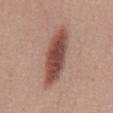The lesion was photographed on a routine skin check and not biopsied; there is no pathology result. The tile uses white-light illumination. This image is a 15 mm lesion crop taken from a total-body photograph. Located on the back. A female patient in their mid- to late 40s. The lesion-visualizer software estimated an outline eccentricity of about 0.95 (0 = round, 1 = elongated) and a symmetry-axis asymmetry near 0.15. The software also gave an automated nevus-likeness rating near 100 out of 100 and a lesion-detection confidence of about 100/100. About 8 mm across.